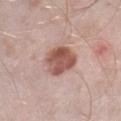Q: Is there a histopathology result?
A: catalogued during a skin exam; not biopsied
Q: Automated lesion metrics?
A: a footprint of about 11 mm², an outline eccentricity of about 0.55 (0 = round, 1 = elongated), and a shape-asymmetry score of about 0.2 (0 = symmetric); a mean CIELAB color near L≈53 a*≈23 b*≈25, roughly 15 lightness units darker than nearby skin, and a lesion-to-skin contrast of about 10 (normalized; higher = more distinct)
Q: How was this image acquired?
A: ~15 mm tile from a whole-body skin photo
Q: Where on the body is the lesion?
A: the right lower leg
Q: Illumination type?
A: white-light
Q: What are the patient's age and sex?
A: male, aged approximately 40
Q: What is the lesion's diameter?
A: about 4 mm